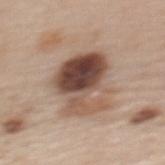Q: What is the lesion's diameter?
A: about 6 mm
Q: Who is the patient?
A: female, aged approximately 50
Q: Where on the body is the lesion?
A: the upper back
Q: How was this image acquired?
A: ~15 mm crop, total-body skin-cancer survey
Q: How was the tile lit?
A: white-light